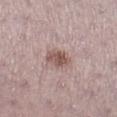Q: Is there a histopathology result?
A: no biopsy performed (imaged during a skin exam)
Q: Patient demographics?
A: female, about 40 years old
Q: Illumination type?
A: white-light
Q: How was this image acquired?
A: ~15 mm crop, total-body skin-cancer survey
Q: How large is the lesion?
A: ~3 mm (longest diameter)
Q: Lesion location?
A: the right lower leg